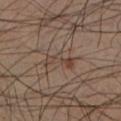Clinical impression:
The lesion was photographed on a routine skin check and not biopsied; there is no pathology result.
Acquisition and patient details:
A male subject approximately 40 years of age. Captured under cross-polarized illumination. This image is a 15 mm lesion crop taken from a total-body photograph. On the leg. The lesion-visualizer software estimated an average lesion color of about L≈41 a*≈15 b*≈24 (CIELAB), a lesion–skin lightness drop of about 7, and a normalized lesion–skin contrast near 6. The analysis additionally found a border-irregularity index near 6/10 and radial color variation of about 0. It also reported a classifier nevus-likeness of about 0/100.